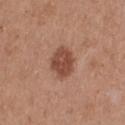TBP lesion metrics: a lesion area of about 8.5 mm², an outline eccentricity of about 0.55 (0 = round, 1 = elongated), and a shape-asymmetry score of about 0.15 (0 = symmetric); an average lesion color of about L≈47 a*≈23 b*≈29 (CIELAB) and roughly 12 lightness units darker than nearby skin | subject: female, aged 38–42 | location: the left thigh | lighting: white-light | size: ≈3.5 mm | image source: ~15 mm crop, total-body skin-cancer survey.